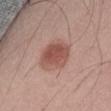The lesion was photographed on a routine skin check and not biopsied; there is no pathology result.
Located on the abdomen.
Imaged with white-light lighting.
The patient is a male approximately 55 years of age.
A roughly 15 mm field-of-view crop from a total-body skin photograph.